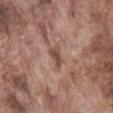Clinical impression: Captured during whole-body skin photography for melanoma surveillance; the lesion was not biopsied. Background: An algorithmic analysis of the crop reported about 10 CIELAB-L* units darker than the surrounding skin and a normalized lesion–skin contrast near 7.5. A 15 mm close-up extracted from a 3D total-body photography capture. Captured under white-light illumination. Located on the abdomen. Measured at roughly 3 mm in maximum diameter. A male patient roughly 75 years of age.